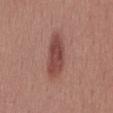The lesion was photographed on a routine skin check and not biopsied; there is no pathology result. The lesion-visualizer software estimated a shape eccentricity near 0.9 and a symmetry-axis asymmetry near 0.15. The analysis additionally found an average lesion color of about L≈46 a*≈24 b*≈24 (CIELAB), roughly 11 lightness units darker than nearby skin, and a normalized lesion–skin contrast near 8.5. The software also gave internal color variation of about 3.5 on a 0–10 scale and peripheral color asymmetry of about 1. A 15 mm close-up tile from a total-body photography series done for melanoma screening. Measured at roughly 6 mm in maximum diameter. A female patient aged 53–57. Imaged with white-light lighting. The lesion is on the mid back.